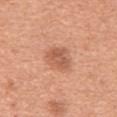Q: Was this lesion biopsied?
A: total-body-photography surveillance lesion; no biopsy
Q: Automated lesion metrics?
A: a lesion color around L≈57 a*≈25 b*≈33 in CIELAB and a normalized lesion–skin contrast near 6.5; a border-irregularity index near 3.5/10, internal color variation of about 2.5 on a 0–10 scale, and peripheral color asymmetry of about 1; a lesion-detection confidence of about 100/100
Q: Where on the body is the lesion?
A: the front of the torso
Q: What lighting was used for the tile?
A: white-light
Q: How large is the lesion?
A: ~3 mm (longest diameter)
Q: Patient demographics?
A: male, in their mid- to late 60s
Q: What is the imaging modality?
A: ~15 mm tile from a whole-body skin photo